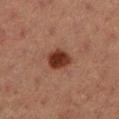| field | value |
|---|---|
| notes | catalogued during a skin exam; not biopsied |
| diameter | ~3 mm (longest diameter) |
| subject | female, roughly 40 years of age |
| site | the leg |
| acquisition | total-body-photography crop, ~15 mm field of view |